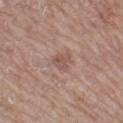notes — imaged on a skin check; not biopsied | image — ~15 mm crop, total-body skin-cancer survey | TBP lesion metrics — a lesion area of about 3.5 mm², a shape eccentricity near 0.65, and two-axis asymmetry of about 0.4; an average lesion color of about L≈52 a*≈20 b*≈24 (CIELAB) and a normalized lesion–skin contrast near 6 | size — ≈2.5 mm | patient — female, roughly 60 years of age | site — the left thigh | illumination — white-light.